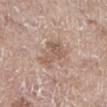workup: no biopsy performed (imaged during a skin exam)
TBP lesion metrics: a lesion color around L≈57 a*≈17 b*≈25 in CIELAB, roughly 9 lightness units darker than nearby skin, and a normalized border contrast of about 6; a border-irregularity index near 5.5/10 and a color-variation rating of about 3.5/10; a classifier nevus-likeness of about 0/100 and lesion-presence confidence of about 100/100
site: the left lower leg
tile lighting: white-light illumination
imaging modality: ~15 mm tile from a whole-body skin photo
patient: female, about 85 years old or older
size: about 5 mm